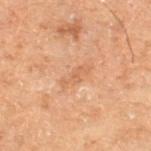biopsy status: no biopsy performed (imaged during a skin exam) | patient: male, aged approximately 70 | anatomic site: the left thigh | image: ~15 mm tile from a whole-body skin photo | diameter: ~3.5 mm (longest diameter).